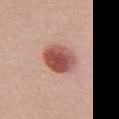follow-up=imaged on a skin check; not biopsied
lesion diameter=~4 mm (longest diameter)
site=the chest
lighting=white-light
subject=female, roughly 60 years of age
image source=~15 mm crop, total-body skin-cancer survey
TBP lesion metrics=a shape eccentricity near 0.6 and two-axis asymmetry of about 0.15